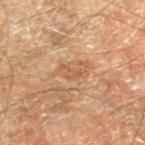Part of a total-body skin-imaging series; this lesion was reviewed on a skin check and was not flagged for biopsy. The recorded lesion diameter is about 2.5 mm. The lesion-visualizer software estimated border irregularity of about 7 on a 0–10 scale, a color-variation rating of about 0/10, and peripheral color asymmetry of about 0. And it measured a classifier nevus-likeness of about 0/100 and a detector confidence of about 100 out of 100 that the crop contains a lesion. Imaged with cross-polarized lighting. A male subject, aged 68–72. The lesion is located on the leg. A 15 mm close-up extracted from a 3D total-body photography capture.